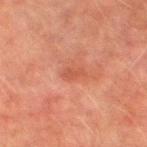No biopsy was performed on this lesion — it was imaged during a full skin examination and was not determined to be concerning.
Located on the left thigh.
Cropped from a whole-body photographic skin survey; the tile spans about 15 mm.
The lesion's longest dimension is about 2.5 mm.
The lesion-visualizer software estimated a lesion area of about 3 mm². And it measured a mean CIELAB color near L≈45 a*≈27 b*≈30, roughly 6 lightness units darker than nearby skin, and a lesion-to-skin contrast of about 5 (normalized; higher = more distinct). The analysis additionally found an automated nevus-likeness rating near 0 out of 100 and a detector confidence of about 100 out of 100 that the crop contains a lesion.
A male subject aged 73–77.
The tile uses cross-polarized illumination.